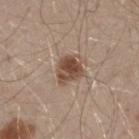biopsy status — no biopsy performed (imaged during a skin exam)
lighting — white-light illumination
acquisition — ~15 mm tile from a whole-body skin photo
site — the mid back
patient — male, aged around 30